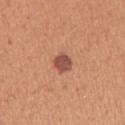This lesion was catalogued during total-body skin photography and was not selected for biopsy. Longest diameter approximately 2.5 mm. A 15 mm close-up extracted from a 3D total-body photography capture. Imaged with white-light lighting. The lesion is on the left upper arm. The subject is a female approximately 40 years of age.